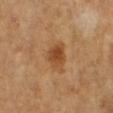biopsy status: catalogued during a skin exam; not biopsied
size: about 4 mm
subject: female, aged around 55
tile lighting: cross-polarized illumination
acquisition: 15 mm crop, total-body photography
automated metrics: a border-irregularity rating of about 2.5/10, a within-lesion color-variation index near 5/10, and a peripheral color-asymmetry measure near 1.5; a classifier nevus-likeness of about 95/100 and lesion-presence confidence of about 100/100
anatomic site: the right lower leg